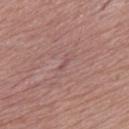Captured during whole-body skin photography for melanoma surveillance; the lesion was not biopsied. A male patient, aged around 75. A roughly 15 mm field-of-view crop from a total-body skin photograph. The lesion is on the upper back.